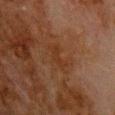Clinical impression:
Part of a total-body skin-imaging series; this lesion was reviewed on a skin check and was not flagged for biopsy.
Image and clinical context:
A male patient aged approximately 80. A 15 mm crop from a total-body photograph taken for skin-cancer surveillance. The tile uses cross-polarized illumination. From the upper back. About 3 mm across.